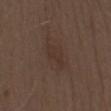notes: total-body-photography surveillance lesion; no biopsy
automated lesion analysis: a lesion area of about 6.5 mm², a shape eccentricity near 0.9, and a shape-asymmetry score of about 0.25 (0 = symmetric)
tile lighting: white-light illumination
size: ~4.5 mm (longest diameter)
subject: male, in their 70s
acquisition: 15 mm crop, total-body photography
site: the chest